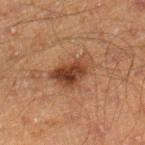Recorded during total-body skin imaging; not selected for excision or biopsy.
Cropped from a whole-body photographic skin survey; the tile spans about 15 mm.
A male subject, aged approximately 45.
On the right lower leg.